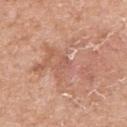Conclusion: On excision, pathology confirmed an intradermal melanocytic nevus.